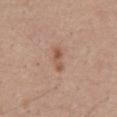Impression:
The lesion was tiled from a total-body skin photograph and was not biopsied.
Acquisition and patient details:
The recorded lesion diameter is about 3.5 mm. A 15 mm close-up extracted from a 3D total-body photography capture. The lesion is on the abdomen. A male subject, aged 53–57.